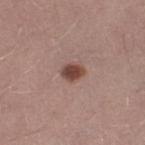Captured during whole-body skin photography for melanoma surveillance; the lesion was not biopsied. Approximately 2.5 mm at its widest. This is a white-light tile. A male subject roughly 30 years of age. Cropped from a total-body skin-imaging series; the visible field is about 15 mm.